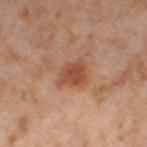biopsy status=imaged on a skin check; not biopsied | tile lighting=cross-polarized | image source=15 mm crop, total-body photography | body site=the leg | subject=female, aged around 55 | diameter=about 3.5 mm.